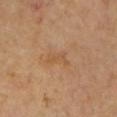<tbp_lesion>
  <image>
    <source>total-body photography crop</source>
    <field_of_view_mm>15</field_of_view_mm>
  </image>
  <patient>
    <sex>male</sex>
    <age_approx>65</age_approx>
  </patient>
  <lesion_size>
    <long_diameter_mm_approx>3.0</long_diameter_mm_approx>
  </lesion_size>
  <site>left upper arm</site>
  <lighting>cross-polarized</lighting>
  <automated_metrics>
    <area_mm2_approx>3.5</area_mm2_approx>
    <eccentricity>0.9</eccentricity>
    <shape_asymmetry>0.45</shape_asymmetry>
    <nevus_likeness_0_100>0</nevus_likeness_0_100>
    <lesion_detection_confidence_0_100>100</lesion_detection_confidence_0_100>
  </automated_metrics>
</tbp_lesion>